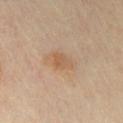{
  "biopsy_status": "not biopsied; imaged during a skin examination",
  "patient": {
    "sex": "female",
    "age_approx": 45
  },
  "image": {
    "source": "total-body photography crop",
    "field_of_view_mm": 15
  },
  "lighting": "cross-polarized",
  "site": "chest"
}